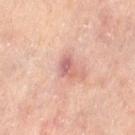Q: Is there a histopathology result?
A: no biopsy performed (imaged during a skin exam)